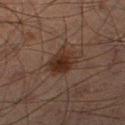Part of a total-body skin-imaging series; this lesion was reviewed on a skin check and was not flagged for biopsy. From the right thigh. A close-up tile cropped from a whole-body skin photograph, about 15 mm across. The subject is a male aged around 70.